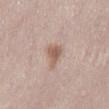Q: Was a biopsy performed?
A: catalogued during a skin exam; not biopsied